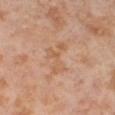The lesion was tiled from a total-body skin photograph and was not biopsied.
This is a cross-polarized tile.
The lesion's longest dimension is about 5 mm.
An algorithmic analysis of the crop reported a lesion color around L≈60 a*≈21 b*≈34 in CIELAB, about 6 CIELAB-L* units darker than the surrounding skin, and a normalized border contrast of about 4.5. The analysis additionally found a within-lesion color-variation index near 2/10 and a peripheral color-asymmetry measure near 0.5.
The lesion is located on the right thigh.
The subject is a female about 55 years old.
Cropped from a whole-body photographic skin survey; the tile spans about 15 mm.